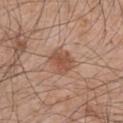Located on the right upper arm.
Cropped from a total-body skin-imaging series; the visible field is about 15 mm.
A male patient aged approximately 45.